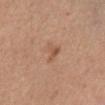Q: Is there a histopathology result?
A: catalogued during a skin exam; not biopsied
Q: Where on the body is the lesion?
A: the left leg
Q: What is the lesion's diameter?
A: ≈3 mm
Q: Automated lesion metrics?
A: a lesion area of about 4 mm² and two-axis asymmetry of about 0.6; about 7 CIELAB-L* units darker than the surrounding skin and a normalized lesion–skin contrast near 5.5; peripheral color asymmetry of about 1; a classifier nevus-likeness of about 10/100 and a detector confidence of about 100 out of 100 that the crop contains a lesion
Q: Who is the patient?
A: male, aged approximately 50
Q: What lighting was used for the tile?
A: cross-polarized
Q: How was this image acquired?
A: ~15 mm tile from a whole-body skin photo